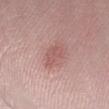biopsy status = imaged on a skin check; not biopsied
subject = male, roughly 50 years of age
location = the arm
imaging modality = total-body-photography crop, ~15 mm field of view
diameter = about 4 mm